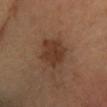Findings:
• workup — imaged on a skin check; not biopsied
• patient — female, in their 40s
• site — the left forearm
• tile lighting — cross-polarized illumination
• image source — ~15 mm crop, total-body skin-cancer survey